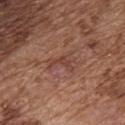Clinical summary: The lesion is located on the chest. An algorithmic analysis of the crop reported a within-lesion color-variation index near 1.5/10 and a peripheral color-asymmetry measure near 0.5. A male patient, in their mid-70s. A 15 mm close-up extracted from a 3D total-body photography capture. This is a white-light tile. Approximately 3.5 mm at its widest.